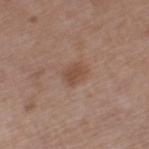The lesion was tiled from a total-body skin photograph and was not biopsied. An algorithmic analysis of the crop reported a footprint of about 4.5 mm² and a symmetry-axis asymmetry near 0.2. It also reported a mean CIELAB color near L≈48 a*≈19 b*≈28, about 8 CIELAB-L* units darker than the surrounding skin, and a lesion-to-skin contrast of about 6.5 (normalized; higher = more distinct). And it measured a nevus-likeness score of about 80/100 and a lesion-detection confidence of about 100/100. The tile uses white-light illumination. Cropped from a whole-body photographic skin survey; the tile spans about 15 mm. A male patient, in their mid- to late 70s. The recorded lesion diameter is about 2.5 mm. The lesion is on the left thigh.